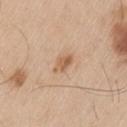Captured during whole-body skin photography for melanoma surveillance; the lesion was not biopsied.
On the left thigh.
A roughly 15 mm field-of-view crop from a total-body skin photograph.
A male patient approximately 60 years of age.
Captured under white-light illumination.
The recorded lesion diameter is about 3 mm.